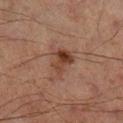No biopsy was performed on this lesion — it was imaged during a full skin examination and was not determined to be concerning.
About 3.5 mm across.
The subject is a male aged 63–67.
Captured under cross-polarized illumination.
The lesion is on the left lower leg.
A 15 mm crop from a total-body photograph taken for skin-cancer surveillance.
Automated image analysis of the tile measured an eccentricity of roughly 0.65. It also reported an average lesion color of about L≈32 a*≈17 b*≈23 (CIELAB), about 8 CIELAB-L* units darker than the surrounding skin, and a normalized border contrast of about 8.